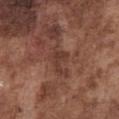{
  "biopsy_status": "not biopsied; imaged during a skin examination",
  "patient": {
    "sex": "male",
    "age_approx": 75
  },
  "image": {
    "source": "total-body photography crop",
    "field_of_view_mm": 15
  },
  "lighting": "white-light",
  "lesion_size": {
    "long_diameter_mm_approx": 2.5
  },
  "site": "chest"
}